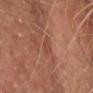The lesion was tiled from a total-body skin photograph and was not biopsied.